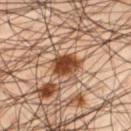Imaged during a routine full-body skin examination; the lesion was not biopsied and no histopathology is available.
The tile uses cross-polarized illumination.
A male subject, in their mid-40s.
The lesion-visualizer software estimated an area of roughly 7 mm² and two-axis asymmetry of about 0.25. The analysis additionally found a mean CIELAB color near L≈42 a*≈22 b*≈32, roughly 17 lightness units darker than nearby skin, and a normalized border contrast of about 13. The analysis additionally found a within-lesion color-variation index near 4/10 and radial color variation of about 1.5. The software also gave a detector confidence of about 100 out of 100 that the crop contains a lesion.
Approximately 3.5 mm at its widest.
A 15 mm close-up extracted from a 3D total-body photography capture.
On the left thigh.